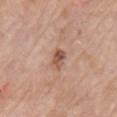Case summary:
• biopsy status · no biopsy performed (imaged during a skin exam)
• body site · the chest
• subject · male, approximately 80 years of age
• acquisition · 15 mm crop, total-body photography